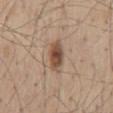notes: total-body-photography surveillance lesion; no biopsy
image source: ~15 mm crop, total-body skin-cancer survey
subject: male, roughly 55 years of age
diameter: about 4 mm
anatomic site: the mid back